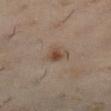Impression:
Recorded during total-body skin imaging; not selected for excision or biopsy.
Image and clinical context:
Cropped from a whole-body photographic skin survey; the tile spans about 15 mm. The total-body-photography lesion software estimated a shape eccentricity near 0.5 and a shape-asymmetry score of about 0.35 (0 = symmetric). A female patient roughly 45 years of age. From the left lower leg.